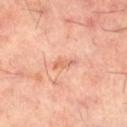biopsy_status: not biopsied; imaged during a skin examination
image:
  source: total-body photography crop
  field_of_view_mm: 15
patient:
  sex: male
  age_approx: 60
site: left thigh